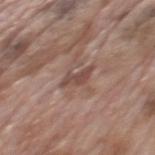Imaged with white-light lighting. The lesion is on the mid back. A male patient, approximately 70 years of age. The lesion's longest dimension is about 3.5 mm. A lesion tile, about 15 mm wide, cut from a 3D total-body photograph.